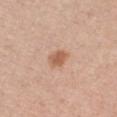Impression: No biopsy was performed on this lesion — it was imaged during a full skin examination and was not determined to be concerning. Context: The lesion's longest dimension is about 2.5 mm. A female subject roughly 40 years of age. Imaged with white-light lighting. The lesion-visualizer software estimated a lesion area of about 4.5 mm², an eccentricity of roughly 0.6, and a symmetry-axis asymmetry near 0.25. The software also gave a lesion color around L≈59 a*≈22 b*≈33 in CIELAB, a lesion–skin lightness drop of about 10, and a lesion-to-skin contrast of about 7.5 (normalized; higher = more distinct). The analysis additionally found an automated nevus-likeness rating near 80 out of 100 and lesion-presence confidence of about 100/100. A 15 mm close-up tile from a total-body photography series done for melanoma screening. On the chest.